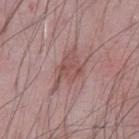Assessment: No biopsy was performed on this lesion — it was imaged during a full skin examination and was not determined to be concerning.